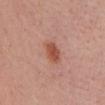{
  "image": {
    "source": "total-body photography crop",
    "field_of_view_mm": 15
  },
  "patient": {
    "sex": "female",
    "age_approx": 50
  },
  "site": "head or neck"
}